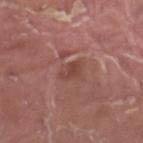workup=catalogued during a skin exam; not biopsied
subject=male, aged 38–42
image=~15 mm crop, total-body skin-cancer survey
lesion size=~2.5 mm (longest diameter)
body site=the left thigh